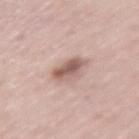notes = no biopsy performed (imaged during a skin exam); subject = female, aged 48–52; acquisition = total-body-photography crop, ~15 mm field of view; anatomic site = the left upper arm.